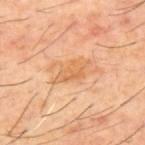workup = no biopsy performed (imaged during a skin exam); image source = 15 mm crop, total-body photography; patient = male, approximately 60 years of age; site = the upper back.